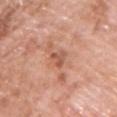Assessment: Part of a total-body skin-imaging series; this lesion was reviewed on a skin check and was not flagged for biopsy. Acquisition and patient details: Located on the right upper arm. A male subject, aged 58 to 62. A close-up tile cropped from a whole-body skin photograph, about 15 mm across. The recorded lesion diameter is about 3 mm. The lesion-visualizer software estimated a lesion area of about 3.5 mm² and a shape-asymmetry score of about 0.45 (0 = symmetric). The analysis additionally found a mean CIELAB color near L≈56 a*≈25 b*≈31, roughly 10 lightness units darker than nearby skin, and a lesion-to-skin contrast of about 6.5 (normalized; higher = more distinct). The analysis additionally found a within-lesion color-variation index near 1/10 and radial color variation of about 0.5.